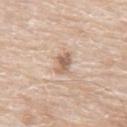Part of a total-body skin-imaging series; this lesion was reviewed on a skin check and was not flagged for biopsy. The subject is a male in their 80s. This is a white-light tile. Approximately 3 mm at its widest. The lesion is located on the abdomen. A lesion tile, about 15 mm wide, cut from a 3D total-body photograph. Automated image analysis of the tile measured a lesion color around L≈62 a*≈17 b*≈29 in CIELAB and roughly 12 lightness units darker than nearby skin.